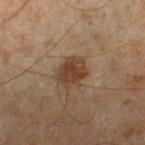No biopsy was performed on this lesion — it was imaged during a full skin examination and was not determined to be concerning.
The lesion is on the right lower leg.
The total-body-photography lesion software estimated a shape eccentricity near 0.6 and a shape-asymmetry score of about 0.2 (0 = symmetric). The software also gave an automated nevus-likeness rating near 80 out of 100 and lesion-presence confidence of about 100/100.
This is a cross-polarized tile.
This image is a 15 mm lesion crop taken from a total-body photograph.
A male patient, in their mid- to late 40s.